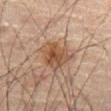Assessment:
No biopsy was performed on this lesion — it was imaged during a full skin examination and was not determined to be concerning.